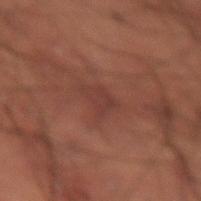Clinical summary:
From the lower back. Imaged with cross-polarized lighting. The lesion's longest dimension is about 3 mm. A male subject, in their 50s. A 15 mm crop from a total-body photograph taken for skin-cancer surveillance.